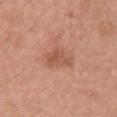<lesion>
  <biopsy_status>not biopsied; imaged during a skin examination</biopsy_status>
  <site>chest</site>
  <image>
    <source>total-body photography crop</source>
    <field_of_view_mm>15</field_of_view_mm>
  </image>
  <lighting>white-light</lighting>
  <automated_metrics>
    <cielab_L>54</cielab_L>
    <cielab_a>24</cielab_a>
    <cielab_b>31</cielab_b>
    <vs_skin_darker_L>9.0</vs_skin_darker_L>
  </automated_metrics>
  <patient>
    <sex>female</sex>
    <age_approx>60</age_approx>
  </patient>
</lesion>